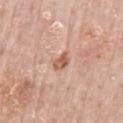Clinical impression: The lesion was photographed on a routine skin check and not biopsied; there is no pathology result. Clinical summary: From the mid back. A roughly 15 mm field-of-view crop from a total-body skin photograph. A male patient, roughly 80 years of age.